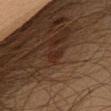Q: How large is the lesion?
A: about 2.5 mm
Q: How was this image acquired?
A: ~15 mm tile from a whole-body skin photo
Q: Lesion location?
A: the chest
Q: Who is the patient?
A: male, aged 58 to 62